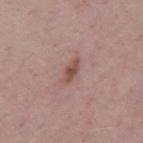Image and clinical context: A 15 mm close-up tile from a total-body photography series done for melanoma screening. A male subject aged 48–52. The lesion is located on the upper back. This is a white-light tile. The lesion's longest dimension is about 3 mm. Automated image analysis of the tile measured a border-irregularity rating of about 3/10, a color-variation rating of about 2.5/10, and peripheral color asymmetry of about 0.5. The software also gave a classifier nevus-likeness of about 0/100 and a lesion-detection confidence of about 100/100.